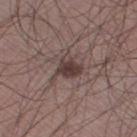Case summary:
– workup · imaged on a skin check; not biopsied
– image · total-body-photography crop, ~15 mm field of view
– tile lighting · white-light illumination
– size · ~4 mm (longest diameter)
– body site · the leg
– patient · male, aged 33 to 37